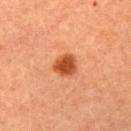Assessment:
This lesion was catalogued during total-body skin photography and was not selected for biopsy.
Image and clinical context:
Captured under cross-polarized illumination. A female subject aged around 60. A lesion tile, about 15 mm wide, cut from a 3D total-body photograph. The recorded lesion diameter is about 2.5 mm. The lesion is on the arm.